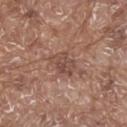biopsy_status: not biopsied; imaged during a skin examination
patient:
  sex: male
  age_approx: 80
automated_metrics:
  border_irregularity_0_10: 3.5
  color_variation_0_10: 3.5
  peripheral_color_asymmetry: 1.0
  nevus_likeness_0_100: 0
image:
  source: total-body photography crop
  field_of_view_mm: 15
site: upper back
lighting: white-light
lesion_size:
  long_diameter_mm_approx: 3.5